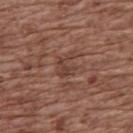Case summary:
- workup · catalogued during a skin exam; not biopsied
- image source · 15 mm crop, total-body photography
- anatomic site · the upper back
- patient · female, aged 73 to 77